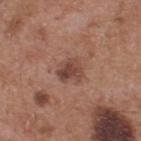Captured during whole-body skin photography for melanoma surveillance; the lesion was not biopsied.
A male patient aged 53–57.
A 15 mm crop from a total-body photograph taken for skin-cancer surveillance.
Automated image analysis of the tile measured a lesion area of about 6 mm², an outline eccentricity of about 0.45 (0 = round, 1 = elongated), and a shape-asymmetry score of about 0.3 (0 = symmetric). It also reported a mean CIELAB color near L≈44 a*≈21 b*≈25, about 10 CIELAB-L* units darker than the surrounding skin, and a normalized lesion–skin contrast near 7.5.
The lesion is on the upper back.
The tile uses white-light illumination.
About 3 mm across.